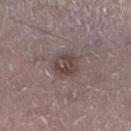Assessment:
Imaged during a routine full-body skin examination; the lesion was not biopsied and no histopathology is available.
Context:
A male subject aged approximately 45. About 3.5 mm across. Cropped from a total-body skin-imaging series; the visible field is about 15 mm. The lesion is located on the leg. Imaged with white-light lighting. An algorithmic analysis of the crop reported a lesion area of about 8.5 mm², an eccentricity of roughly 0.45, and two-axis asymmetry of about 0.15. The analysis additionally found a border-irregularity index near 1.5/10, a within-lesion color-variation index near 5/10, and radial color variation of about 2.